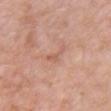A male patient aged 48–52.
The lesion is on the chest.
Imaged with white-light lighting.
Measured at roughly 3 mm in maximum diameter.
A 15 mm crop from a total-body photograph taken for skin-cancer surveillance.